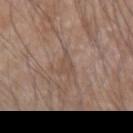follow-up: total-body-photography surveillance lesion; no biopsy
diameter: about 2.5 mm
subject: male, in their mid-70s
imaging modality: ~15 mm tile from a whole-body skin photo
body site: the right forearm